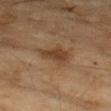Clinical impression:
Captured during whole-body skin photography for melanoma surveillance; the lesion was not biopsied.
Background:
About 4.5 mm across. A roughly 15 mm field-of-view crop from a total-body skin photograph. A female subject, aged approximately 60. The tile uses cross-polarized illumination. Automated image analysis of the tile measured border irregularity of about 4.5 on a 0–10 scale, a within-lesion color-variation index near 2.5/10, and peripheral color asymmetry of about 1. And it measured a lesion-detection confidence of about 100/100. On the left forearm.